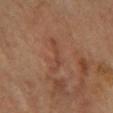{
  "biopsy_status": "not biopsied; imaged during a skin examination",
  "image": {
    "source": "total-body photography crop",
    "field_of_view_mm": 15
  },
  "lesion_size": {
    "long_diameter_mm_approx": 4.5
  },
  "site": "mid back",
  "patient": {
    "sex": "male",
    "age_approx": 60
  }
}